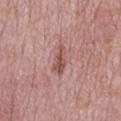{"biopsy_status": "not biopsied; imaged during a skin examination", "site": "mid back", "patient": {"sex": "male", "age_approx": 75}, "image": {"source": "total-body photography crop", "field_of_view_mm": 15}}